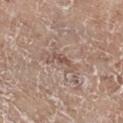Impression: Captured during whole-body skin photography for melanoma surveillance; the lesion was not biopsied. Context: The recorded lesion diameter is about 3.5 mm. The lesion is located on the left lower leg. The tile uses white-light illumination. A 15 mm close-up extracted from a 3D total-body photography capture. A female patient aged approximately 75.